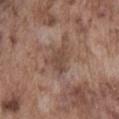Assessment:
The lesion was photographed on a routine skin check and not biopsied; there is no pathology result.
Acquisition and patient details:
A male subject, aged approximately 75. Cropped from a total-body skin-imaging series; the visible field is about 15 mm. The total-body-photography lesion software estimated a shape eccentricity near 0.7 and two-axis asymmetry of about 0.4. The analysis additionally found border irregularity of about 4.5 on a 0–10 scale and a within-lesion color-variation index near 1.5/10. From the abdomen. This is a white-light tile. Longest diameter approximately 4 mm.